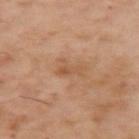The lesion was tiled from a total-body skin photograph and was not biopsied.
A lesion tile, about 15 mm wide, cut from a 3D total-body photograph.
Measured at roughly 3 mm in maximum diameter.
Captured under cross-polarized illumination.
A male patient, approximately 55 years of age.
Located on the arm.